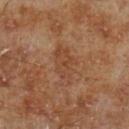Captured during whole-body skin photography for melanoma surveillance; the lesion was not biopsied.